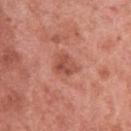- biopsy status · imaged on a skin check; not biopsied
- subject · female, aged 48 to 52
- lighting · white-light illumination
- location · the arm
- image source · ~15 mm tile from a whole-body skin photo
- diameter · ~3 mm (longest diameter)
- image-analysis metrics · a mean CIELAB color near L≈51 a*≈28 b*≈30; a border-irregularity index near 3/10 and a peripheral color-asymmetry measure near 2; a classifier nevus-likeness of about 20/100 and a detector confidence of about 100 out of 100 that the crop contains a lesion